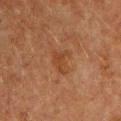Q: How was this image acquired?
A: total-body-photography crop, ~15 mm field of view
Q: Lesion location?
A: the chest
Q: What is the lesion's diameter?
A: ≈3 mm
Q: Illumination type?
A: cross-polarized
Q: Who is the patient?
A: male, aged 58 to 62